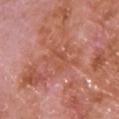| key | value |
|---|---|
| follow-up | no biopsy performed (imaged during a skin exam) |
| imaging modality | ~15 mm tile from a whole-body skin photo |
| anatomic site | the chest |
| TBP lesion metrics | an area of roughly 6.5 mm², an outline eccentricity of about 0.9 (0 = round, 1 = elongated), and a symmetry-axis asymmetry near 0.45; an automated nevus-likeness rating near 0 out of 100 and a lesion-detection confidence of about 80/100 |
| subject | male, aged around 65 |
| illumination | white-light |